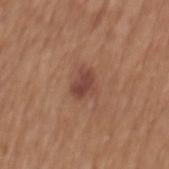Clinical impression: The lesion was photographed on a routine skin check and not biopsied; there is no pathology result. Acquisition and patient details: The lesion is located on the back. The tile uses white-light illumination. Cropped from a total-body skin-imaging series; the visible field is about 15 mm. The patient is a male in their mid- to late 60s.